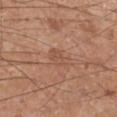Clinical impression:
Captured during whole-body skin photography for melanoma surveillance; the lesion was not biopsied.
Background:
A 15 mm close-up extracted from a 3D total-body photography capture. The total-body-photography lesion software estimated a lesion area of about 4.5 mm², an outline eccentricity of about 0.65 (0 = round, 1 = elongated), and a shape-asymmetry score of about 0.25 (0 = symmetric). The analysis additionally found an average lesion color of about L≈52 a*≈21 b*≈30 (CIELAB), about 6 CIELAB-L* units darker than the surrounding skin, and a normalized border contrast of about 4.5. The lesion is located on the leg. The recorded lesion diameter is about 2.5 mm. A male patient aged approximately 55.